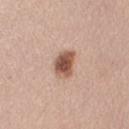notes: catalogued during a skin exam; not biopsied
illumination: white-light illumination
image: ~15 mm crop, total-body skin-cancer survey
subject: female, about 45 years old
image-analysis metrics: an outline eccentricity of about 0.75 (0 = round, 1 = elongated); about 17 CIELAB-L* units darker than the surrounding skin and a lesion-to-skin contrast of about 11 (normalized; higher = more distinct); a border-irregularity rating of about 2.5/10, internal color variation of about 4 on a 0–10 scale, and a peripheral color-asymmetry measure near 1; a classifier nevus-likeness of about 100/100 and lesion-presence confidence of about 100/100
lesion diameter: about 3.5 mm
anatomic site: the right upper arm